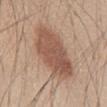Recorded during total-body skin imaging; not selected for excision or biopsy.
A region of skin cropped from a whole-body photographic capture, roughly 15 mm wide.
The recorded lesion diameter is about 7.5 mm.
A male patient aged approximately 45.
Automated tile analysis of the lesion measured a lesion-detection confidence of about 100/100.
This is a white-light tile.
On the abdomen.